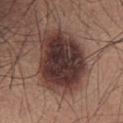Part of a total-body skin-imaging series; this lesion was reviewed on a skin check and was not flagged for biopsy.
Imaged with white-light lighting.
The total-body-photography lesion software estimated a lesion color around L≈33 a*≈18 b*≈19 in CIELAB. It also reported border irregularity of about 2 on a 0–10 scale, a color-variation rating of about 6/10, and radial color variation of about 2. It also reported an automated nevus-likeness rating near 25 out of 100 and a lesion-detection confidence of about 100/100.
This image is a 15 mm lesion crop taken from a total-body photograph.
A male patient aged 23–27.
Longest diameter approximately 7.5 mm.
On the abdomen.